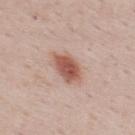Q: Was this lesion biopsied?
A: imaged on a skin check; not biopsied
Q: Automated lesion metrics?
A: an area of roughly 9 mm², an outline eccentricity of about 0.8 (0 = round, 1 = elongated), and a shape-asymmetry score of about 0.2 (0 = symmetric); roughly 13 lightness units darker than nearby skin and a lesion-to-skin contrast of about 9 (normalized; higher = more distinct); a border-irregularity rating of about 2/10, a color-variation rating of about 4/10, and radial color variation of about 1; an automated nevus-likeness rating near 100 out of 100 and lesion-presence confidence of about 100/100
Q: Lesion location?
A: the upper back
Q: Who is the patient?
A: male, aged approximately 50
Q: How large is the lesion?
A: ~5 mm (longest diameter)
Q: What kind of image is this?
A: ~15 mm crop, total-body skin-cancer survey